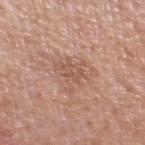Q: What is the anatomic site?
A: the left lower leg
Q: How was the tile lit?
A: white-light illumination
Q: What is the imaging modality?
A: total-body-photography crop, ~15 mm field of view
Q: What is the lesion's diameter?
A: ≈2.5 mm
Q: Automated lesion metrics?
A: an average lesion color of about L≈54 a*≈21 b*≈28 (CIELAB), roughly 7 lightness units darker than nearby skin, and a normalized border contrast of about 5; a border-irregularity index near 8.5/10, a within-lesion color-variation index near 0/10, and radial color variation of about 0; a classifier nevus-likeness of about 0/100 and a detector confidence of about 95 out of 100 that the crop contains a lesion
Q: What are the patient's age and sex?
A: male, aged 78–82